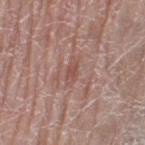Imaged during a routine full-body skin examination; the lesion was not biopsied and no histopathology is available. Imaged with white-light lighting. Longest diameter approximately 2.5 mm. A 15 mm close-up tile from a total-body photography series done for melanoma screening. The subject is a female aged 58 to 62. The lesion is located on the left thigh. Automated tile analysis of the lesion measured a lesion area of about 2.5 mm² and an eccentricity of roughly 0.9. And it measured a border-irregularity index near 2.5/10, internal color variation of about 0.5 on a 0–10 scale, and peripheral color asymmetry of about 0.